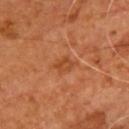Captured during whole-body skin photography for melanoma surveillance; the lesion was not biopsied.
A male patient, aged approximately 55.
From the chest.
A 15 mm close-up extracted from a 3D total-body photography capture.